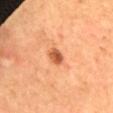Q: How was the tile lit?
A: cross-polarized illumination
Q: What did automated image analysis measure?
A: an area of roughly 3.5 mm², an outline eccentricity of about 0.5 (0 = round, 1 = elongated), and a shape-asymmetry score of about 0.15 (0 = symmetric); a mean CIELAB color near L≈54 a*≈29 b*≈39, roughly 14 lightness units darker than nearby skin, and a lesion-to-skin contrast of about 9 (normalized; higher = more distinct); a lesion-detection confidence of about 100/100
Q: What is the imaging modality?
A: total-body-photography crop, ~15 mm field of view
Q: Patient demographics?
A: female, aged 53 to 57
Q: Lesion location?
A: the mid back